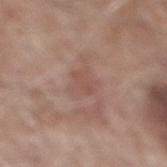biopsy status: catalogued during a skin exam; not biopsied